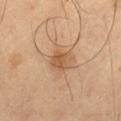Background:
The recorded lesion diameter is about 3 mm. The total-body-photography lesion software estimated a lesion area of about 6 mm² and an outline eccentricity of about 0.35 (0 = round, 1 = elongated). The analysis additionally found a lesion-detection confidence of about 100/100. The patient is a male in their 50s. The lesion is on the left thigh. A 15 mm crop from a total-body photograph taken for skin-cancer surveillance. The tile uses cross-polarized illumination.